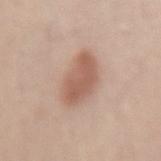Acquisition and patient details: About 5 mm across. The patient is a male roughly 55 years of age. A region of skin cropped from a whole-body photographic capture, roughly 15 mm wide. The lesion-visualizer software estimated a shape eccentricity near 0.75. It also reported an average lesion color of about L≈58 a*≈20 b*≈28 (CIELAB) and a lesion-to-skin contrast of about 7.5 (normalized; higher = more distinct). Located on the back. Imaged with white-light lighting.